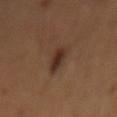workup: imaged on a skin check; not biopsied
imaging modality: ~15 mm crop, total-body skin-cancer survey
subject: female
location: the mid back